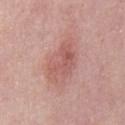Acquisition and patient details:
Longest diameter approximately 5.5 mm. The subject is a male about 55 years old. The lesion is on the abdomen. A 15 mm close-up extracted from a 3D total-body photography capture.